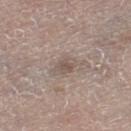Captured during whole-body skin photography for melanoma surveillance; the lesion was not biopsied. A roughly 15 mm field-of-view crop from a total-body skin photograph. The total-body-photography lesion software estimated an area of roughly 3.5 mm², a shape eccentricity near 0.8, and two-axis asymmetry of about 0.25. The analysis additionally found a lesion color around L≈53 a*≈13 b*≈21 in CIELAB. It also reported a border-irregularity index near 2.5/10 and a within-lesion color-variation index near 1.5/10. Imaged with white-light lighting. The patient is a male about 80 years old. From the left thigh. Longest diameter approximately 3 mm.